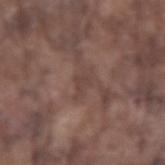{"biopsy_status": "not biopsied; imaged during a skin examination", "lesion_size": {"long_diameter_mm_approx": 3.0}, "image": {"source": "total-body photography crop", "field_of_view_mm": 15}, "patient": {"sex": "male", "age_approx": 75}, "site": "right forearm", "automated_metrics": {"border_irregularity_0_10": 5.5, "color_variation_0_10": 0.5, "peripheral_color_asymmetry": 0.0, "nevus_likeness_0_100": 0, "lesion_detection_confidence_0_100": 80}}